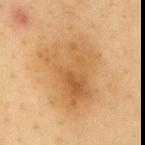The lesion was tiled from a total-body skin photograph and was not biopsied. The lesion is on the mid back. Longest diameter approximately 8.5 mm. This is a cross-polarized tile. Cropped from a whole-body photographic skin survey; the tile spans about 15 mm. An algorithmic analysis of the crop reported a mean CIELAB color near L≈52 a*≈17 b*≈37. The analysis additionally found a border-irregularity rating of about 3.5/10, a color-variation rating of about 5.5/10, and peripheral color asymmetry of about 2. And it measured a classifier nevus-likeness of about 10/100. A female patient approximately 40 years of age.